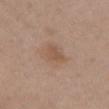| key | value |
|---|---|
| notes | total-body-photography surveillance lesion; no biopsy |
| lighting | white-light illumination |
| acquisition | 15 mm crop, total-body photography |
| automated metrics | two-axis asymmetry of about 0.35; an average lesion color of about L≈53 a*≈18 b*≈29 (CIELAB); a border-irregularity index near 3.5/10, internal color variation of about 2.5 on a 0–10 scale, and peripheral color asymmetry of about 1; a nevus-likeness score of about 5/100 and lesion-presence confidence of about 100/100 |
| lesion diameter | ~3 mm (longest diameter) |
| patient | female, in their mid-60s |
| location | the abdomen |